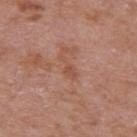Assessment: Imaged during a routine full-body skin examination; the lesion was not biopsied and no histopathology is available. Background: The patient is a male aged 68–72. On the right upper arm. A 15 mm close-up extracted from a 3D total-body photography capture. The total-body-photography lesion software estimated a lesion color around L≈51 a*≈23 b*≈30 in CIELAB and a normalized border contrast of about 5.5. The tile uses white-light illumination.